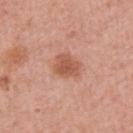The lesion was photographed on a routine skin check and not biopsied; there is no pathology result. Longest diameter approximately 3.5 mm. The total-body-photography lesion software estimated a mean CIELAB color near L≈56 a*≈26 b*≈32, roughly 10 lightness units darker than nearby skin, and a lesion-to-skin contrast of about 7.5 (normalized; higher = more distinct). And it measured a border-irregularity index near 2.5/10, internal color variation of about 3 on a 0–10 scale, and peripheral color asymmetry of about 1. And it measured a nevus-likeness score of about 85/100 and lesion-presence confidence of about 100/100. A 15 mm crop from a total-body photograph taken for skin-cancer surveillance. Imaged with white-light lighting. The patient is a female aged 58–62. The lesion is located on the right upper arm.